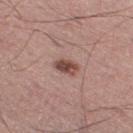Impression:
No biopsy was performed on this lesion — it was imaged during a full skin examination and was not determined to be concerning.
Clinical summary:
Measured at roughly 3 mm in maximum diameter. Automated tile analysis of the lesion measured a lesion area of about 4.5 mm², a shape eccentricity near 0.75, and a symmetry-axis asymmetry near 0.25. It also reported an average lesion color of about L≈48 a*≈21 b*≈23 (CIELAB), roughly 13 lightness units darker than nearby skin, and a lesion-to-skin contrast of about 9.5 (normalized; higher = more distinct). The analysis additionally found radial color variation of about 1. The analysis additionally found a classifier nevus-likeness of about 90/100 and lesion-presence confidence of about 100/100. Located on the right thigh. This is a white-light tile. A male patient, in their 60s. This image is a 15 mm lesion crop taken from a total-body photograph.